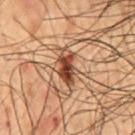Notes:
– biopsy status — catalogued during a skin exam; not biopsied
– acquisition — ~15 mm tile from a whole-body skin photo
– illumination — cross-polarized
– patient — male, aged 48 to 52
– TBP lesion metrics — an area of roughly 7 mm², a shape eccentricity near 0.85, and two-axis asymmetry of about 0.25; a mean CIELAB color near L≈35 a*≈19 b*≈26 and a normalized border contrast of about 11.5; a border-irregularity rating of about 3/10 and internal color variation of about 6.5 on a 0–10 scale; a classifier nevus-likeness of about 65/100 and a lesion-detection confidence of about 100/100
– diameter — ≈4 mm
– body site — the mid back